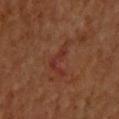Q: What kind of image is this?
A: total-body-photography crop, ~15 mm field of view
Q: What did automated image analysis measure?
A: an eccentricity of roughly 0.9 and a shape-asymmetry score of about 0.8 (0 = symmetric); a detector confidence of about 100 out of 100 that the crop contains a lesion
Q: Lesion location?
A: the upper back
Q: What lighting was used for the tile?
A: cross-polarized illumination
Q: Patient demographics?
A: male, aged around 60
Q: What is the lesion's diameter?
A: about 4.5 mm